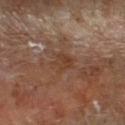| key | value |
|---|---|
| notes | catalogued during a skin exam; not biopsied |
| anatomic site | the right forearm |
| acquisition | total-body-photography crop, ~15 mm field of view |
| subject | male, aged approximately 65 |